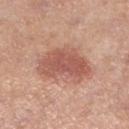| key | value |
|---|---|
| notes | catalogued during a skin exam; not biopsied |
| anatomic site | the left lower leg |
| patient | female, about 40 years old |
| size | about 6.5 mm |
| lighting | white-light |
| image | ~15 mm crop, total-body skin-cancer survey |